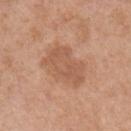This lesion was catalogued during total-body skin photography and was not selected for biopsy.